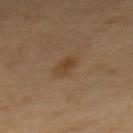biopsy status: total-body-photography surveillance lesion; no biopsy | acquisition: ~15 mm tile from a whole-body skin photo | anatomic site: the mid back | lesion diameter: ≈2.5 mm | subject: male, about 60 years old | automated lesion analysis: an outline eccentricity of about 0.75 (0 = round, 1 = elongated) and a shape-asymmetry score of about 0.2 (0 = symmetric); an average lesion color of about L≈39 a*≈16 b*≈31 (CIELAB) and roughly 7 lightness units darker than nearby skin; border irregularity of about 2 on a 0–10 scale and a within-lesion color-variation index near 2.5/10; a nevus-likeness score of about 45/100 and a lesion-detection confidence of about 100/100.